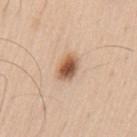This lesion was catalogued during total-body skin photography and was not selected for biopsy. A 15 mm crop from a total-body photograph taken for skin-cancer surveillance. The lesion's longest dimension is about 3 mm. The lesion is located on the left upper arm. A male subject approximately 60 years of age. The tile uses white-light illumination.